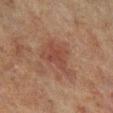<record>
<biopsy_status>not biopsied; imaged during a skin examination</biopsy_status>
<image>
  <source>total-body photography crop</source>
  <field_of_view_mm>15</field_of_view_mm>
</image>
<site>right lower leg</site>
<lighting>cross-polarized</lighting>
<patient>
  <sex>male</sex>
  <age_approx>70</age_approx>
</patient>
<lesion_size>
  <long_diameter_mm_approx>4.5</long_diameter_mm_approx>
</lesion_size>
</record>